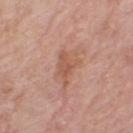biopsy status: catalogued during a skin exam; not biopsied | acquisition: ~15 mm tile from a whole-body skin photo | illumination: white-light illumination | anatomic site: the leg | lesion size: ~4 mm (longest diameter) | subject: female, roughly 70 years of age | automated lesion analysis: an eccentricity of roughly 0.75 and a shape-asymmetry score of about 0.45 (0 = symmetric); border irregularity of about 5.5 on a 0–10 scale, internal color variation of about 2.5 on a 0–10 scale, and radial color variation of about 1; an automated nevus-likeness rating near 0 out of 100 and a detector confidence of about 100 out of 100 that the crop contains a lesion.